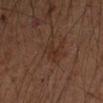Clinical impression: Recorded during total-body skin imaging; not selected for excision or biopsy. Context: Longest diameter approximately 2.5 mm. From the left forearm. The total-body-photography lesion software estimated an automated nevus-likeness rating near 0 out of 100. A male patient aged around 50. A roughly 15 mm field-of-view crop from a total-body skin photograph.